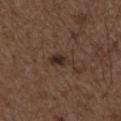Q: Was a biopsy performed?
A: no biopsy performed (imaged during a skin exam)
Q: What lighting was used for the tile?
A: white-light
Q: Patient demographics?
A: male, aged around 50
Q: What did automated image analysis measure?
A: a shape eccentricity near 0.85 and two-axis asymmetry of about 0.3; a lesion color around L≈29 a*≈15 b*≈21 in CIELAB and a normalized lesion–skin contrast near 8.5
Q: What is the imaging modality?
A: 15 mm crop, total-body photography
Q: Lesion location?
A: the right upper arm